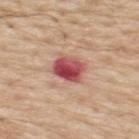Assessment: Part of a total-body skin-imaging series; this lesion was reviewed on a skin check and was not flagged for biopsy. Image and clinical context: Captured under white-light illumination. The lesion's longest dimension is about 3.5 mm. The lesion is located on the upper back. A 15 mm close-up extracted from a 3D total-body photography capture. Automated image analysis of the tile measured two-axis asymmetry of about 0.15. And it measured a lesion color around L≈51 a*≈33 b*≈24 in CIELAB, about 17 CIELAB-L* units darker than the surrounding skin, and a normalized lesion–skin contrast near 12. And it measured an automated nevus-likeness rating near 0 out of 100 and a detector confidence of about 100 out of 100 that the crop contains a lesion. A male patient about 70 years old.